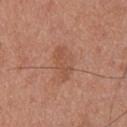This lesion was catalogued during total-body skin photography and was not selected for biopsy.
A male patient, aged approximately 30.
A 15 mm close-up extracted from a 3D total-body photography capture.
The lesion is on the back.
This is a white-light tile.
The recorded lesion diameter is about 4 mm.